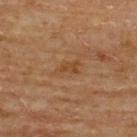From the upper back. A male patient aged around 65. Automated image analysis of the tile measured about 6 CIELAB-L* units darker than the surrounding skin and a normalized border contrast of about 6. The analysis additionally found radial color variation of about 0.5. The analysis additionally found a nevus-likeness score of about 5/100 and a detector confidence of about 100 out of 100 that the crop contains a lesion. This is a cross-polarized tile. A 15 mm close-up extracted from a 3D total-body photography capture.